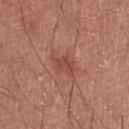Q: Is there a histopathology result?
A: total-body-photography surveillance lesion; no biopsy
Q: What lighting was used for the tile?
A: white-light
Q: What did automated image analysis measure?
A: a lesion area of about 4 mm² and a shape-asymmetry score of about 0.35 (0 = symmetric); a lesion-to-skin contrast of about 6 (normalized; higher = more distinct); a border-irregularity rating of about 3.5/10 and radial color variation of about 0.5; a classifier nevus-likeness of about 5/100 and a lesion-detection confidence of about 100/100
Q: Lesion size?
A: about 3 mm
Q: How was this image acquired?
A: ~15 mm crop, total-body skin-cancer survey
Q: What is the anatomic site?
A: the right lower leg
Q: What are the patient's age and sex?
A: male, aged approximately 70